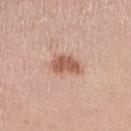No biopsy was performed on this lesion — it was imaged during a full skin examination and was not determined to be concerning. The lesion is on the right lower leg. The patient is a female aged around 65. This image is a 15 mm lesion crop taken from a total-body photograph.